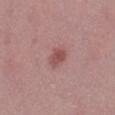workup: imaged on a skin check; not biopsied
subject: female, aged around 45
lesion diameter: about 2.5 mm
site: the right thigh
tile lighting: white-light illumination
imaging modality: ~15 mm tile from a whole-body skin photo